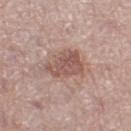Context:
The lesion is on the right thigh. A close-up tile cropped from a whole-body skin photograph, about 15 mm across. Measured at roughly 4.5 mm in maximum diameter. The total-body-photography lesion software estimated a lesion area of about 12 mm², a shape eccentricity near 0.6, and a shape-asymmetry score of about 0.3 (0 = symmetric). The software also gave a border-irregularity index near 3.5/10, a within-lesion color-variation index near 3.5/10, and radial color variation of about 1. It also reported a nevus-likeness score of about 15/100 and lesion-presence confidence of about 100/100. The patient is a female aged 63 to 67. This is a white-light tile.